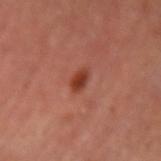| key | value |
|---|---|
| notes | imaged on a skin check; not biopsied |
| location | the left upper arm |
| diameter | about 2.5 mm |
| imaging modality | ~15 mm tile from a whole-body skin photo |
| subject | male, roughly 65 years of age |
| automated metrics | an automated nevus-likeness rating near 100 out of 100 and a detector confidence of about 100 out of 100 that the crop contains a lesion |
| tile lighting | cross-polarized illumination |